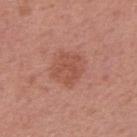The lesion was photographed on a routine skin check and not biopsied; there is no pathology result.
From the right upper arm.
A female patient, aged 48 to 52.
A 15 mm crop from a total-body photograph taken for skin-cancer surveillance.